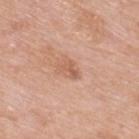follow-up = imaged on a skin check; not biopsied | subject = male, roughly 80 years of age | location = the upper back | image = 15 mm crop, total-body photography.